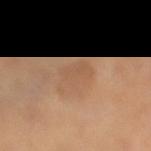Impression:
This lesion was catalogued during total-body skin photography and was not selected for biopsy.
Image and clinical context:
A female subject, aged around 65. On the leg. This image is a 15 mm lesion crop taken from a total-body photograph.